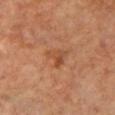Assessment: The lesion was photographed on a routine skin check and not biopsied; there is no pathology result. Background: A close-up tile cropped from a whole-body skin photograph, about 15 mm across. A female patient aged around 60. On the chest. Automated image analysis of the tile measured a footprint of about 4.5 mm², a shape eccentricity near 0.45, and a shape-asymmetry score of about 0.45 (0 = symmetric). The software also gave an average lesion color of about L≈45 a*≈23 b*≈34 (CIELAB) and about 7 CIELAB-L* units darker than the surrounding skin. The software also gave a border-irregularity rating of about 4/10, internal color variation of about 4 on a 0–10 scale, and radial color variation of about 1.5. Imaged with cross-polarized lighting.